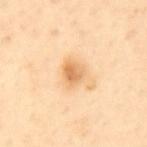Notes:
– follow-up — no biopsy performed (imaged during a skin exam)
– imaging modality — 15 mm crop, total-body photography
– illumination — cross-polarized illumination
– diameter — ~3 mm (longest diameter)
– subject — male, aged 63–67
– anatomic site — the mid back